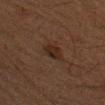Impression: The lesion was tiled from a total-body skin photograph and was not biopsied. Context: A male patient, aged 48–52. A 15 mm close-up tile from a total-body photography series done for melanoma screening. The lesion is located on the left upper arm.